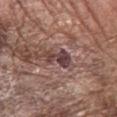Captured during whole-body skin photography for melanoma surveillance; the lesion was not biopsied. A 15 mm close-up extracted from a 3D total-body photography capture. Automated image analysis of the tile measured an outline eccentricity of about 0.85 (0 = round, 1 = elongated) and a shape-asymmetry score of about 0.45 (0 = symmetric). It also reported a lesion color around L≈41 a*≈20 b*≈18 in CIELAB, roughly 12 lightness units darker than nearby skin, and a normalized border contrast of about 10. A male subject, aged 68–72. On the left forearm.